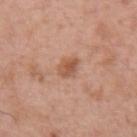Captured during whole-body skin photography for melanoma surveillance; the lesion was not biopsied.
A female subject, aged approximately 40.
The tile uses white-light illumination.
On the arm.
The lesion's longest dimension is about 2.5 mm.
A 15 mm close-up tile from a total-body photography series done for melanoma screening.
Automated tile analysis of the lesion measured an average lesion color of about L≈54 a*≈23 b*≈32 (CIELAB), a lesion–skin lightness drop of about 10, and a lesion-to-skin contrast of about 7 (normalized; higher = more distinct). The analysis additionally found an automated nevus-likeness rating near 65 out of 100 and lesion-presence confidence of about 100/100.